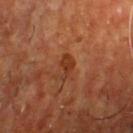Assessment:
This lesion was catalogued during total-body skin photography and was not selected for biopsy.
Background:
Cropped from a whole-body photographic skin survey; the tile spans about 15 mm. The patient is a male about 55 years old. Imaged with cross-polarized lighting. Located on the chest. Measured at roughly 3 mm in maximum diameter.